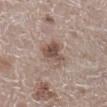Clinical impression: The lesion was photographed on a routine skin check and not biopsied; there is no pathology result.